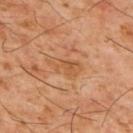• notes: catalogued during a skin exam; not biopsied
• lesion size: ≈4 mm
• patient: male, aged approximately 60
• illumination: cross-polarized
• imaging modality: ~15 mm crop, total-body skin-cancer survey
• body site: the back